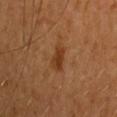The lesion was photographed on a routine skin check and not biopsied; there is no pathology result. A female patient, about 55 years old. This image is a 15 mm lesion crop taken from a total-body photograph. Automated image analysis of the tile measured a lesion area of about 5 mm², an eccentricity of roughly 0.85, and a shape-asymmetry score of about 0.35 (0 = symmetric). The analysis additionally found an average lesion color of about L≈39 a*≈23 b*≈36 (CIELAB) and roughly 8 lightness units darker than nearby skin. Located on the back.